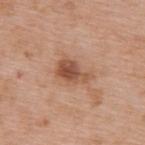Assessment: Imaged during a routine full-body skin examination; the lesion was not biopsied and no histopathology is available.